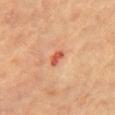workup=no biopsy performed (imaged during a skin exam).